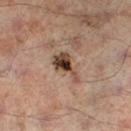Imaged during a routine full-body skin examination; the lesion was not biopsied and no histopathology is available.
The lesion is located on the left lower leg.
Cropped from a whole-body photographic skin survey; the tile spans about 15 mm.
A male subject, about 65 years old.
Approximately 4 mm at its widest.
The lesion-visualizer software estimated a lesion area of about 7 mm² and a shape-asymmetry score of about 0.5 (0 = symmetric). The analysis additionally found an average lesion color of about L≈42 a*≈16 b*≈26 (CIELAB), about 15 CIELAB-L* units darker than the surrounding skin, and a normalized lesion–skin contrast near 11. The software also gave a border-irregularity rating of about 5/10, a within-lesion color-variation index near 7/10, and a peripheral color-asymmetry measure near 2. And it measured an automated nevus-likeness rating near 95 out of 100 and lesion-presence confidence of about 100/100.